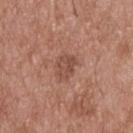Case summary:
* follow-up: no biopsy performed (imaged during a skin exam)
* diameter: ≈2.5 mm
* TBP lesion metrics: an area of roughly 5 mm², an outline eccentricity of about 0.65 (0 = round, 1 = elongated), and two-axis asymmetry of about 0.2; a border-irregularity rating of about 2/10, internal color variation of about 3 on a 0–10 scale, and peripheral color asymmetry of about 1; an automated nevus-likeness rating near 5 out of 100 and a detector confidence of about 100 out of 100 that the crop contains a lesion
* patient: male, roughly 55 years of age
* lighting: white-light illumination
* image: total-body-photography crop, ~15 mm field of view
* site: the upper back